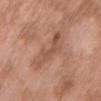| field | value |
|---|---|
| lesion size | ≈6.5 mm |
| automated lesion analysis | a peripheral color-asymmetry measure near 1.5 |
| anatomic site | the chest |
| lighting | white-light |
| imaging modality | ~15 mm tile from a whole-body skin photo |
| patient | female, aged 68–72 |